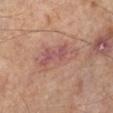Findings:
- biopsy status — total-body-photography surveillance lesion; no biopsy
- image source — 15 mm crop, total-body photography
- body site — the left lower leg
- subject — male, aged 63 to 67